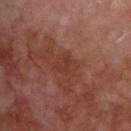Clinical impression:
Captured during whole-body skin photography for melanoma surveillance; the lesion was not biopsied.
Background:
Captured under cross-polarized illumination. A male patient, aged around 70. Longest diameter approximately 3.5 mm. On the upper back. This image is a 15 mm lesion crop taken from a total-body photograph.